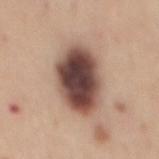This is a white-light tile.
A male subject approximately 50 years of age.
A roughly 15 mm field-of-view crop from a total-body skin photograph.
The lesion is located on the mid back.
An algorithmic analysis of the crop reported a lesion color around L≈46 a*≈19 b*≈23 in CIELAB, roughly 22 lightness units darker than nearby skin, and a normalized border contrast of about 15.5. The software also gave a border-irregularity rating of about 1.5/10, a within-lesion color-variation index near 9/10, and a peripheral color-asymmetry measure near 2.5.